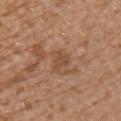notes: no biopsy performed (imaged during a skin exam); illumination: white-light; patient: female, roughly 30 years of age; location: the chest; imaging modality: 15 mm crop, total-body photography; lesion diameter: ~3 mm (longest diameter).